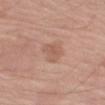No biopsy was performed on this lesion — it was imaged during a full skin examination and was not determined to be concerning.
Measured at roughly 3 mm in maximum diameter.
A region of skin cropped from a whole-body photographic capture, roughly 15 mm wide.
Located on the arm.
Automated image analysis of the tile measured roughly 7 lightness units darker than nearby skin and a normalized border contrast of about 5. It also reported a nevus-likeness score of about 0/100 and a detector confidence of about 100 out of 100 that the crop contains a lesion.
The subject is a male approximately 60 years of age.
The tile uses white-light illumination.